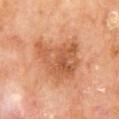follow-up = total-body-photography surveillance lesion; no biopsy
image-analysis metrics = a lesion area of about 22 mm², a shape eccentricity near 0.65, and two-axis asymmetry of about 0.35; a nevus-likeness score of about 0/100 and a detector confidence of about 100 out of 100 that the crop contains a lesion
subject = male, approximately 70 years of age
image = total-body-photography crop, ~15 mm field of view
lighting = cross-polarized illumination
lesion size = ~6 mm (longest diameter)
location = the mid back